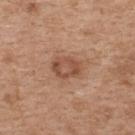A 15 mm crop from a total-body photograph taken for skin-cancer surveillance.
A female subject, about 40 years old.
Located on the back.
This is a white-light tile.